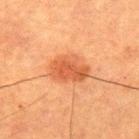Imaged during a routine full-body skin examination; the lesion was not biopsied and no histopathology is available.
The total-body-photography lesion software estimated a mean CIELAB color near L≈47 a*≈26 b*≈35, about 9 CIELAB-L* units darker than the surrounding skin, and a normalized lesion–skin contrast near 7.
The patient is a male aged around 50.
Measured at roughly 4.5 mm in maximum diameter.
A 15 mm close-up tile from a total-body photography series done for melanoma screening.
The tile uses cross-polarized illumination.
The lesion is located on the mid back.